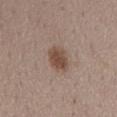Clinical summary: The lesion is located on the mid back. A female subject about 45 years old. Automated image analysis of the tile measured a classifier nevus-likeness of about 95/100. Captured under white-light illumination. A close-up tile cropped from a whole-body skin photograph, about 15 mm across.